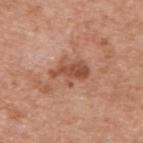Case summary:
• workup — no biopsy performed (imaged during a skin exam)
• automated metrics — a lesion color around L≈51 a*≈24 b*≈32 in CIELAB, a lesion–skin lightness drop of about 11, and a normalized lesion–skin contrast near 8; a border-irregularity index near 4.5/10 and peripheral color asymmetry of about 1.5
• patient — male, in their 60s
• body site — the upper back
• size — ~4.5 mm (longest diameter)
• illumination — white-light
• imaging modality — ~15 mm crop, total-body skin-cancer survey